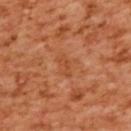Findings:
* follow-up — catalogued during a skin exam; not biopsied